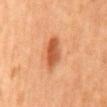The lesion was photographed on a routine skin check and not biopsied; there is no pathology result. Imaged with cross-polarized lighting. This image is a 15 mm lesion crop taken from a total-body photograph. A male subject, approximately 65 years of age. About 5 mm across. On the mid back.